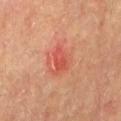Assessment:
Recorded during total-body skin imaging; not selected for excision or biopsy.
Background:
The subject is a male about 75 years old. Captured under cross-polarized illumination. An algorithmic analysis of the crop reported a footprint of about 5 mm², an eccentricity of roughly 0.85, and a shape-asymmetry score of about 0.3 (0 = symmetric). It also reported an average lesion color of about L≈44 a*≈31 b*≈29 (CIELAB), a lesion–skin lightness drop of about 7, and a normalized border contrast of about 6. It also reported a border-irregularity rating of about 3.5/10, a color-variation rating of about 3.5/10, and a peripheral color-asymmetry measure near 1.5. The analysis additionally found a nevus-likeness score of about 0/100 and lesion-presence confidence of about 100/100. Located on the mid back. A region of skin cropped from a whole-body photographic capture, roughly 15 mm wide. The lesion's longest dimension is about 3.5 mm.